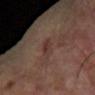<tbp_lesion>
  <biopsy_status>not biopsied; imaged during a skin examination</biopsy_status>
  <automated_metrics>
    <cielab_L>32</cielab_L>
    <cielab_a>18</cielab_a>
    <cielab_b>19</cielab_b>
    <vs_skin_darker_L>6.0</vs_skin_darker_L>
    <vs_skin_contrast_norm>6.0</vs_skin_contrast_norm>
    <border_irregularity_0_10>3.0</border_irregularity_0_10>
    <color_variation_0_10>2.0</color_variation_0_10>
    <peripheral_color_asymmetry>0.5</peripheral_color_asymmetry>
  </automated_metrics>
  <site>right forearm</site>
  <image>
    <source>total-body photography crop</source>
    <field_of_view_mm>15</field_of_view_mm>
  </image>
  <patient>
    <sex>male</sex>
    <age_approx>65</age_approx>
  </patient>
  <lesion_size>
    <long_diameter_mm_approx>3.0</long_diameter_mm_approx>
  </lesion_size>
  <lighting>cross-polarized</lighting>
</tbp_lesion>